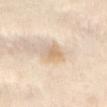This lesion was catalogued during total-body skin photography and was not selected for biopsy. The patient is a female aged around 55. Imaged with cross-polarized lighting. A 15 mm crop from a total-body photograph taken for skin-cancer surveillance. Automated image analysis of the tile measured an area of roughly 3.5 mm², an outline eccentricity of about 0.8 (0 = round, 1 = elongated), and two-axis asymmetry of about 0.3. The analysis additionally found an average lesion color of about L≈71 a*≈14 b*≈34 (CIELAB), a lesion–skin lightness drop of about 10, and a lesion-to-skin contrast of about 6.5 (normalized; higher = more distinct). It also reported an automated nevus-likeness rating near 5 out of 100 and a lesion-detection confidence of about 75/100. The lesion is on the abdomen.